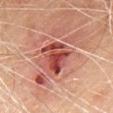notes: no biopsy performed (imaged during a skin exam)
TBP lesion metrics: a lesion color around L≈49 a*≈35 b*≈30 in CIELAB, roughly 14 lightness units darker than nearby skin, and a lesion-to-skin contrast of about 9.5 (normalized; higher = more distinct)
imaging modality: 15 mm crop, total-body photography
lesion size: ~4 mm (longest diameter)
patient: female, aged 68 to 72
anatomic site: the chest
lighting: white-light